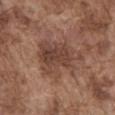The lesion was tiled from a total-body skin photograph and was not biopsied. Located on the abdomen. Cropped from a total-body skin-imaging series; the visible field is about 15 mm. The subject is a male in their mid-70s. About 6.5 mm across.